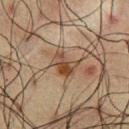Recorded during total-body skin imaging; not selected for excision or biopsy.
A male patient, aged approximately 60.
This is a cross-polarized tile.
The lesion is on the chest.
This image is a 15 mm lesion crop taken from a total-body photograph.
Automated tile analysis of the lesion measured a lesion-to-skin contrast of about 8.5 (normalized; higher = more distinct). And it measured a border-irregularity rating of about 3.5/10, a within-lesion color-variation index near 7.5/10, and a peripheral color-asymmetry measure near 2.5. The analysis additionally found a classifier nevus-likeness of about 30/100 and a detector confidence of about 100 out of 100 that the crop contains a lesion.
Measured at roughly 3 mm in maximum diameter.